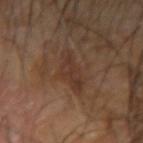The lesion is located on the left forearm. A 15 mm crop from a total-body photograph taken for skin-cancer surveillance. A male patient approximately 65 years of age.